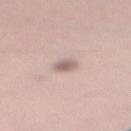Part of a total-body skin-imaging series; this lesion was reviewed on a skin check and was not flagged for biopsy.